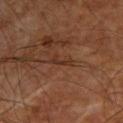Q: Was a biopsy performed?
A: catalogued during a skin exam; not biopsied
Q: Lesion location?
A: the right upper arm
Q: What did automated image analysis measure?
A: a mean CIELAB color near L≈31 a*≈20 b*≈28 and a lesion–skin lightness drop of about 6
Q: What is the lesion's diameter?
A: ~2.5 mm (longest diameter)
Q: What are the patient's age and sex?
A: aged 63–67
Q: What is the imaging modality?
A: total-body-photography crop, ~15 mm field of view